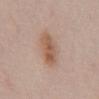Recorded during total-body skin imaging; not selected for excision or biopsy. From the mid back. A 15 mm close-up extracted from a 3D total-body photography capture. A male subject, about 55 years old. Imaged with white-light lighting. An algorithmic analysis of the crop reported a lesion area of about 11 mm² and an eccentricity of roughly 0.9. Measured at roughly 5.5 mm in maximum diameter.